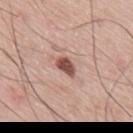A 15 mm crop from a total-body photograph taken for skin-cancer surveillance. The patient is a male roughly 75 years of age. The lesion is on the upper back. The lesion's longest dimension is about 2.5 mm.